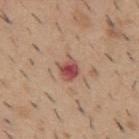Q: Was this lesion biopsied?
A: no biopsy performed (imaged during a skin exam)
Q: How was this image acquired?
A: ~15 mm crop, total-body skin-cancer survey
Q: What are the patient's age and sex?
A: male, roughly 40 years of age
Q: Lesion size?
A: about 2.5 mm
Q: What did automated image analysis measure?
A: an outline eccentricity of about 0.3 (0 = round, 1 = elongated) and two-axis asymmetry of about 0.3; a mean CIELAB color near L≈50 a*≈26 b*≈25 and a normalized lesion–skin contrast near 9; border irregularity of about 3 on a 0–10 scale, a within-lesion color-variation index near 4.5/10, and radial color variation of about 1; a nevus-likeness score of about 0/100 and lesion-presence confidence of about 100/100
Q: Where on the body is the lesion?
A: the mid back